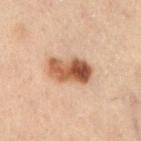The lesion was photographed on a routine skin check and not biopsied; there is no pathology result. Measured at roughly 5.5 mm in maximum diameter. The lesion is on the left lower leg. This image is a 15 mm lesion crop taken from a total-body photograph. The tile uses cross-polarized illumination. The subject is a male in their mid- to late 50s.